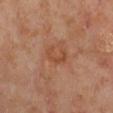Q: Is there a histopathology result?
A: catalogued during a skin exam; not biopsied